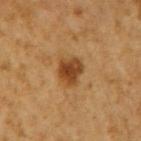No biopsy was performed on this lesion — it was imaged during a full skin examination and was not determined to be concerning. This is a cross-polarized tile. The lesion is located on the right upper arm. Cropped from a whole-body photographic skin survey; the tile spans about 15 mm. A male patient, about 60 years old. The lesion-visualizer software estimated a lesion color around L≈40 a*≈20 b*≈36 in CIELAB.